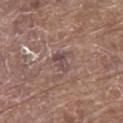follow-up: imaged on a skin check; not biopsied
tile lighting: white-light
subject: male, about 80 years old
acquisition: ~15 mm crop, total-body skin-cancer survey
site: the upper back
diameter: ~2.5 mm (longest diameter)